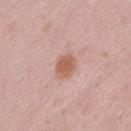<record>
<biopsy_status>not biopsied; imaged during a skin examination</biopsy_status>
<patient>
  <sex>female</sex>
  <age_approx>30</age_approx>
</patient>
<lighting>white-light</lighting>
<image>
  <source>total-body photography crop</source>
  <field_of_view_mm>15</field_of_view_mm>
</image>
<automated_metrics>
  <area_mm2_approx>5.0</area_mm2_approx>
  <eccentricity>0.7</eccentricity>
  <shape_asymmetry>0.2</shape_asymmetry>
  <cielab_L>58</cielab_L>
  <cielab_a>23</cielab_a>
  <cielab_b>29</cielab_b>
  <vs_skin_contrast_norm>8.0</vs_skin_contrast_norm>
</automated_metrics>
<site>leg</site>
</record>